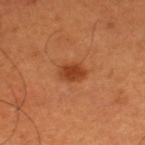Part of a total-body skin-imaging series; this lesion was reviewed on a skin check and was not flagged for biopsy. From the left thigh. A male patient aged around 50. A roughly 15 mm field-of-view crop from a total-body skin photograph. Longest diameter approximately 3 mm. The tile uses cross-polarized illumination.